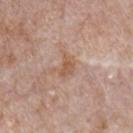Notes:
• biopsy status · total-body-photography surveillance lesion; no biopsy
• anatomic site · the front of the torso
• patient · male, aged approximately 70
• size · ~3 mm (longest diameter)
• imaging modality · total-body-photography crop, ~15 mm field of view